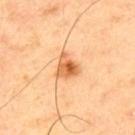The lesion was photographed on a routine skin check and not biopsied; there is no pathology result.
The recorded lesion diameter is about 3 mm.
A male patient, roughly 40 years of age.
On the upper back.
A region of skin cropped from a whole-body photographic capture, roughly 15 mm wide.